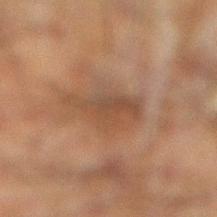Q: Was this lesion biopsied?
A: imaged on a skin check; not biopsied
Q: What are the patient's age and sex?
A: male, in their 50s
Q: Lesion size?
A: ~5.5 mm (longest diameter)
Q: Illumination type?
A: cross-polarized
Q: Where on the body is the lesion?
A: the right lower leg
Q: What is the imaging modality?
A: ~15 mm tile from a whole-body skin photo